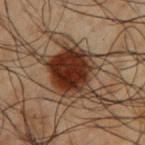Assessment: No biopsy was performed on this lesion — it was imaged during a full skin examination and was not determined to be concerning. Image and clinical context: A male patient aged approximately 50. Imaged with cross-polarized lighting. The lesion-visualizer software estimated a nevus-likeness score of about 90/100 and lesion-presence confidence of about 100/100. The lesion is on the left upper arm. The lesion's longest dimension is about 10.5 mm. A lesion tile, about 15 mm wide, cut from a 3D total-body photograph.